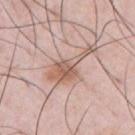follow-up: imaged on a skin check; not biopsied
subject: male, aged 33 to 37
body site: the chest
tile lighting: white-light
diameter: ~6 mm (longest diameter)
TBP lesion metrics: an area of roughly 9.5 mm² and a shape eccentricity near 0.9; border irregularity of about 7 on a 0–10 scale, a within-lesion color-variation index near 4.5/10, and peripheral color asymmetry of about 1.5
image: 15 mm crop, total-body photography